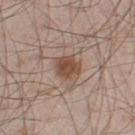Impression: Part of a total-body skin-imaging series; this lesion was reviewed on a skin check and was not flagged for biopsy. Acquisition and patient details: The lesion is on the left thigh. Cropped from a total-body skin-imaging series; the visible field is about 15 mm. The patient is a male aged around 60.